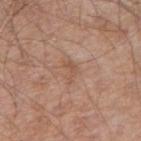{
  "biopsy_status": "not biopsied; imaged during a skin examination",
  "automated_metrics": {
    "shape_asymmetry": 0.55,
    "border_irregularity_0_10": 5.5,
    "color_variation_0_10": 0.0,
    "peripheral_color_asymmetry": 0.0
  },
  "lighting": "white-light",
  "site": "right upper arm",
  "patient": {
    "sex": "male",
    "age_approx": 65
  },
  "image": {
    "source": "total-body photography crop",
    "field_of_view_mm": 15
  }
}